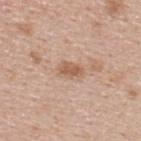follow-up=catalogued during a skin exam; not biopsied | illumination=white-light | site=the back | imaging modality=15 mm crop, total-body photography | diameter=about 3 mm | subject=male, aged 38–42.